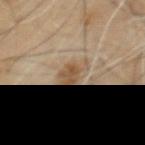| field | value |
|---|---|
| follow-up | total-body-photography surveillance lesion; no biopsy |
| image source | ~15 mm tile from a whole-body skin photo |
| subject | male, aged approximately 60 |
| body site | the mid back |